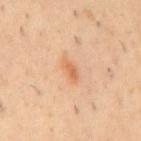• notes · no biopsy performed (imaged during a skin exam)
• subject · male, aged around 55
• diameter · ≈3 mm
• illumination · cross-polarized illumination
• imaging modality · 15 mm crop, total-body photography
• image-analysis metrics · an area of roughly 3.5 mm² and a symmetry-axis asymmetry near 0.3; internal color variation of about 2.5 on a 0–10 scale and peripheral color asymmetry of about 1
• body site · the mid back